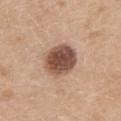Q: Was a biopsy performed?
A: imaged on a skin check; not biopsied
Q: What lighting was used for the tile?
A: white-light illumination
Q: Lesion location?
A: the upper back
Q: What did automated image analysis measure?
A: a mean CIELAB color near L≈50 a*≈19 b*≈27, a lesion–skin lightness drop of about 18, and a normalized border contrast of about 12; internal color variation of about 5 on a 0–10 scale and radial color variation of about 1.5; a classifier nevus-likeness of about 60/100
Q: Who is the patient?
A: female, approximately 60 years of age
Q: Lesion size?
A: about 4.5 mm
Q: What is the imaging modality?
A: total-body-photography crop, ~15 mm field of view